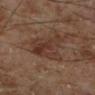Q: Is there a histopathology result?
A: imaged on a skin check; not biopsied
Q: Who is the patient?
A: male, in their 70s
Q: Lesion location?
A: the leg
Q: How was this image acquired?
A: ~15 mm tile from a whole-body skin photo